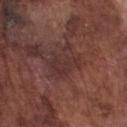biopsy status: total-body-photography surveillance lesion; no biopsy
body site: the front of the torso
image source: total-body-photography crop, ~15 mm field of view
lesion size: about 4 mm
TBP lesion metrics: a footprint of about 10 mm², an eccentricity of roughly 0.65, and two-axis asymmetry of about 0.3; a mean CIELAB color near L≈33 a*≈20 b*≈20, roughly 6 lightness units darker than nearby skin, and a lesion-to-skin contrast of about 6 (normalized; higher = more distinct); internal color variation of about 3 on a 0–10 scale and peripheral color asymmetry of about 1; an automated nevus-likeness rating near 0 out of 100 and lesion-presence confidence of about 70/100
illumination: white-light illumination
patient: male, approximately 75 years of age